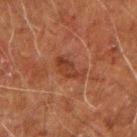A male subject, roughly 60 years of age.
Cropped from a whole-body photographic skin survey; the tile spans about 15 mm.
Approximately 4 mm at its widest.
The tile uses cross-polarized illumination.
From the left arm.